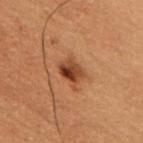biopsy status = no biopsy performed (imaged during a skin exam)
diameter = ≈2.5 mm
acquisition = ~15 mm crop, total-body skin-cancer survey
TBP lesion metrics = a border-irregularity index near 2/10 and a peripheral color-asymmetry measure near 3; a classifier nevus-likeness of about 85/100 and a detector confidence of about 100 out of 100 that the crop contains a lesion
anatomic site = the arm
lighting = cross-polarized
patient = male, aged approximately 50